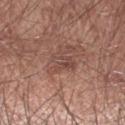<record>
  <biopsy_status>not biopsied; imaged during a skin examination</biopsy_status>
  <patient>
    <sex>male</sex>
    <age_approx>65</age_approx>
  </patient>
  <lighting>white-light</lighting>
  <image>
    <source>total-body photography crop</source>
    <field_of_view_mm>15</field_of_view_mm>
  </image>
  <site>arm</site>
  <automated_metrics>
    <cielab_L>46</cielab_L>
    <cielab_a>20</cielab_a>
    <cielab_b>24</cielab_b>
    <vs_skin_contrast_norm>5.5</vs_skin_contrast_norm>
  </automated_metrics>
</record>